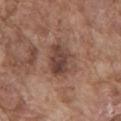Q: Was a biopsy performed?
A: catalogued during a skin exam; not biopsied
Q: Who is the patient?
A: male, in their mid-70s
Q: How was the tile lit?
A: white-light
Q: Lesion size?
A: ~5 mm (longest diameter)
Q: Lesion location?
A: the mid back
Q: What is the imaging modality?
A: 15 mm crop, total-body photography